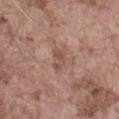Clinical impression: Imaged during a routine full-body skin examination; the lesion was not biopsied and no histopathology is available. Background: Imaged with white-light lighting. A roughly 15 mm field-of-view crop from a total-body skin photograph. On the abdomen. The patient is a male aged around 75. Automated tile analysis of the lesion measured an area of roughly 4.5 mm², an outline eccentricity of about 0.8 (0 = round, 1 = elongated), and two-axis asymmetry of about 0.4. And it measured a mean CIELAB color near L≈51 a*≈19 b*≈25 and about 7 CIELAB-L* units darker than the surrounding skin. The analysis additionally found a border-irregularity rating of about 4.5/10, a color-variation rating of about 1/10, and a peripheral color-asymmetry measure near 0.5. It also reported a nevus-likeness score of about 0/100 and a detector confidence of about 100 out of 100 that the crop contains a lesion. Approximately 3 mm at its widest.